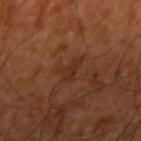{
  "automated_metrics": {
    "area_mm2_approx": 4.5,
    "shape_asymmetry": 0.5,
    "border_irregularity_0_10": 5.0,
    "color_variation_0_10": 1.5,
    "lesion_detection_confidence_0_100": 100
  },
  "lesion_size": {
    "long_diameter_mm_approx": 3.0
  },
  "image": {
    "source": "total-body photography crop",
    "field_of_view_mm": 15
  },
  "patient": {
    "age_approx": 65
  },
  "site": "right upper arm"
}